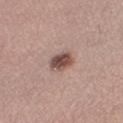notes: total-body-photography surveillance lesion; no biopsy
subject: female, aged 23–27
body site: the leg
TBP lesion metrics: a lesion area of about 6 mm², an outline eccentricity of about 0.45 (0 = round, 1 = elongated), and a shape-asymmetry score of about 0.15 (0 = symmetric); an average lesion color of about L≈49 a*≈19 b*≈22 (CIELAB); a border-irregularity index near 1.5/10 and a within-lesion color-variation index near 4/10
tile lighting: white-light
image: ~15 mm tile from a whole-body skin photo
diameter: ≈3 mm